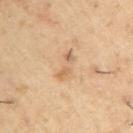notes = total-body-photography surveillance lesion; no biopsy
imaging modality = ~15 mm tile from a whole-body skin photo
tile lighting = cross-polarized
anatomic site = the right upper arm
subject = male, about 55 years old
size = ≈3.5 mm